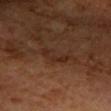Longest diameter approximately 4 mm.
A 15 mm close-up extracted from a 3D total-body photography capture.
From the left forearm.
The lesion-visualizer software estimated a lesion color around L≈25 a*≈18 b*≈24 in CIELAB, roughly 5 lightness units darker than nearby skin, and a normalized lesion–skin contrast near 6.
The subject is a male approximately 60 years of age.